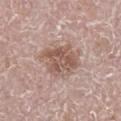The lesion is on the left leg. A close-up tile cropped from a whole-body skin photograph, about 15 mm across. Captured under white-light illumination. The patient is a male aged 68–72. Approximately 5.5 mm at its widest.